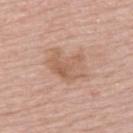The lesion was photographed on a routine skin check and not biopsied; there is no pathology result. The lesion is located on the upper back. Cropped from a whole-body photographic skin survey; the tile spans about 15 mm. A male patient, aged around 65.